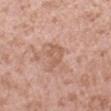{"biopsy_status": "not biopsied; imaged during a skin examination", "lighting": "white-light", "image": {"source": "total-body photography crop", "field_of_view_mm": 15}, "patient": {"sex": "female", "age_approx": 40}, "site": "left forearm", "automated_metrics": {"area_mm2_approx": 5.0, "shape_asymmetry": 0.3, "border_irregularity_0_10": 3.5, "color_variation_0_10": 2.0, "nevus_likeness_0_100": 0, "lesion_detection_confidence_0_100": 100}, "lesion_size": {"long_diameter_mm_approx": 3.5}}